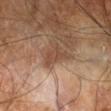{
  "biopsy_status": "not biopsied; imaged during a skin examination",
  "lesion_size": {
    "long_diameter_mm_approx": 3.5
  },
  "site": "right leg",
  "patient": {
    "sex": "male",
    "age_approx": 60
  },
  "image": {
    "source": "total-body photography crop",
    "field_of_view_mm": 15
  }
}